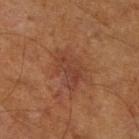workup = total-body-photography surveillance lesion; no biopsy | acquisition = 15 mm crop, total-body photography | site = the left leg | subject = male, aged 63 to 67.